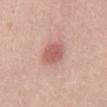Assessment:
Captured during whole-body skin photography for melanoma surveillance; the lesion was not biopsied.
Image and clinical context:
Captured under white-light illumination. A 15 mm crop from a total-body photograph taken for skin-cancer surveillance. About 3 mm across. A female subject about 55 years old. The lesion is located on the abdomen.